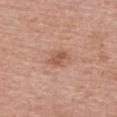<tbp_lesion>
  <biopsy_status>not biopsied; imaged during a skin examination</biopsy_status>
  <site>front of the torso</site>
  <patient>
    <sex>female</sex>
    <age_approx>65</age_approx>
  </patient>
  <automated_metrics>
    <area_mm2_approx>4.5</area_mm2_approx>
    <eccentricity>0.75</eccentricity>
    <shape_asymmetry>0.3</shape_asymmetry>
    <border_irregularity_0_10>2.5</border_irregularity_0_10>
    <nevus_likeness_0_100>5</nevus_likeness_0_100>
    <lesion_detection_confidence_0_100>100</lesion_detection_confidence_0_100>
  </automated_metrics>
  <lighting>white-light</lighting>
  <lesion_size>
    <long_diameter_mm_approx>3.0</long_diameter_mm_approx>
  </lesion_size>
  <image>
    <source>total-body photography crop</source>
    <field_of_view_mm>15</field_of_view_mm>
  </image>
</tbp_lesion>